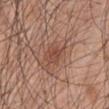The lesion was photographed on a routine skin check and not biopsied; there is no pathology result.
This is a white-light tile.
The lesion is located on the left upper arm.
This image is a 15 mm lesion crop taken from a total-body photograph.
A male subject, in their mid- to late 40s.
Automated tile analysis of the lesion measured a lesion area of about 5.5 mm², an eccentricity of roughly 0.8, and a shape-asymmetry score of about 0.25 (0 = symmetric). The software also gave an average lesion color of about L≈48 a*≈22 b*≈28 (CIELAB), about 8 CIELAB-L* units darker than the surrounding skin, and a lesion-to-skin contrast of about 6.5 (normalized; higher = more distinct).
Approximately 4 mm at its widest.